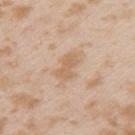Q: Was a biopsy performed?
A: catalogued during a skin exam; not biopsied
Q: Where on the body is the lesion?
A: the left upper arm
Q: Who is the patient?
A: female, in their mid-20s
Q: What is the imaging modality?
A: ~15 mm tile from a whole-body skin photo